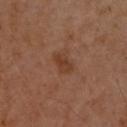Imaged during a routine full-body skin examination; the lesion was not biopsied and no histopathology is available.
Approximately 2.5 mm at its widest.
Located on the upper back.
The patient is a male aged 43–47.
A lesion tile, about 15 mm wide, cut from a 3D total-body photograph.